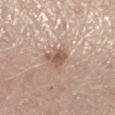workup: catalogued during a skin exam; not biopsied
site: the left lower leg
TBP lesion metrics: an area of roughly 5 mm²; a border-irregularity index near 4/10, a within-lesion color-variation index near 3/10, and radial color variation of about 1; a nevus-likeness score of about 45/100
image source: ~15 mm tile from a whole-body skin photo
tile lighting: white-light
lesion size: ~3 mm (longest diameter)
patient: female, aged approximately 40